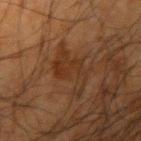Q: Was this lesion biopsied?
A: no biopsy performed (imaged during a skin exam)
Q: Automated lesion metrics?
A: a shape-asymmetry score of about 0.5 (0 = symmetric); a lesion color around L≈26 a*≈17 b*≈26 in CIELAB and a normalized lesion–skin contrast near 6; border irregularity of about 7.5 on a 0–10 scale and a peripheral color-asymmetry measure near 0.5
Q: What lighting was used for the tile?
A: cross-polarized illumination
Q: Lesion location?
A: the right upper arm
Q: How large is the lesion?
A: ~5 mm (longest diameter)
Q: What are the patient's age and sex?
A: male, in their mid- to late 60s
Q: What kind of image is this?
A: ~15 mm crop, total-body skin-cancer survey